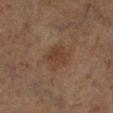| field | value |
|---|---|
| notes | total-body-photography surveillance lesion; no biopsy |
| location | the left lower leg |
| lesion size | about 3 mm |
| patient | male, in their mid-70s |
| acquisition | ~15 mm tile from a whole-body skin photo |
| image-analysis metrics | a nevus-likeness score of about 5/100 and lesion-presence confidence of about 100/100 |